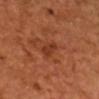This lesion was catalogued during total-body skin photography and was not selected for biopsy.
The lesion's longest dimension is about 3 mm.
From the head or neck.
A lesion tile, about 15 mm wide, cut from a 3D total-body photograph.
A male patient, in their 50s.
An algorithmic analysis of the crop reported a nevus-likeness score of about 0/100 and lesion-presence confidence of about 100/100.